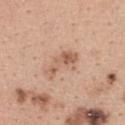notes: no biopsy performed (imaged during a skin exam); illumination: white-light illumination; image-analysis metrics: a shape eccentricity near 0.9; acquisition: ~15 mm tile from a whole-body skin photo; lesion diameter: ~4.5 mm (longest diameter); subject: female, roughly 40 years of age; anatomic site: the upper back.